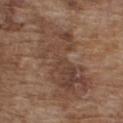The lesion was photographed on a routine skin check and not biopsied; there is no pathology result. Measured at roughly 11.5 mm in maximum diameter. The lesion is located on the chest. A 15 mm close-up extracted from a 3D total-body photography capture. Imaged with white-light lighting. A male subject in their mid- to late 70s. An algorithmic analysis of the crop reported a lesion area of about 40 mm², an eccentricity of roughly 0.9, and a symmetry-axis asymmetry near 0.35. The software also gave an average lesion color of about L≈43 a*≈17 b*≈26 (CIELAB), about 8 CIELAB-L* units darker than the surrounding skin, and a normalized border contrast of about 6.5. And it measured a within-lesion color-variation index near 5.5/10 and peripheral color asymmetry of about 2. The analysis additionally found lesion-presence confidence of about 80/100.